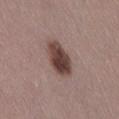– biopsy status · no biopsy performed (imaged during a skin exam)
– automated metrics · an area of roughly 11 mm², an eccentricity of roughly 0.75, and a shape-asymmetry score of about 0.15 (0 = symmetric); border irregularity of about 2 on a 0–10 scale, internal color variation of about 5.5 on a 0–10 scale, and a peripheral color-asymmetry measure near 2
– subject · female, aged approximately 45
– tile lighting · white-light illumination
– imaging modality · ~15 mm crop, total-body skin-cancer survey
– body site · the right thigh